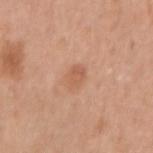No biopsy was performed on this lesion — it was imaged during a full skin examination and was not determined to be concerning. Cropped from a total-body skin-imaging series; the visible field is about 15 mm. On the right upper arm. A female patient, aged around 50.